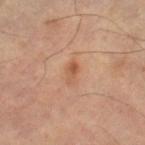Impression:
Captured during whole-body skin photography for melanoma surveillance; the lesion was not biopsied.
Background:
The recorded lesion diameter is about 3 mm. A 15 mm close-up extracted from a 3D total-body photography capture. The total-body-photography lesion software estimated a footprint of about 3 mm², an eccentricity of roughly 0.9, and two-axis asymmetry of about 0.2. Imaged with cross-polarized lighting. The lesion is located on the right thigh.